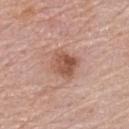The lesion was tiled from a total-body skin photograph and was not biopsied.
On the back.
The lesion's longest dimension is about 3.5 mm.
An algorithmic analysis of the crop reported a lesion color around L≈54 a*≈22 b*≈29 in CIELAB and a normalized lesion–skin contrast near 8. It also reported a border-irregularity index near 2/10, a within-lesion color-variation index near 6/10, and a peripheral color-asymmetry measure near 2.5.
This image is a 15 mm lesion crop taken from a total-body photograph.
A female patient, in their mid- to late 60s.